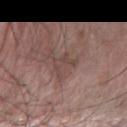This lesion was catalogued during total-body skin photography and was not selected for biopsy.
The lesion-visualizer software estimated lesion-presence confidence of about 95/100.
The patient is a male roughly 75 years of age.
About 3 mm across.
Imaged with white-light lighting.
A close-up tile cropped from a whole-body skin photograph, about 15 mm across.
The lesion is located on the right forearm.